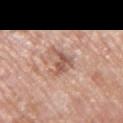Clinical impression: Captured during whole-body skin photography for melanoma surveillance; the lesion was not biopsied. Image and clinical context: Cropped from a whole-body photographic skin survey; the tile spans about 15 mm. A male subject, aged 68–72. The lesion's longest dimension is about 4.5 mm. On the arm.